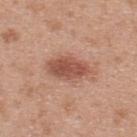notes = catalogued during a skin exam; not biopsied
patient = male, aged 28 to 32
automated metrics = a lesion color around L≈52 a*≈24 b*≈29 in CIELAB, about 12 CIELAB-L* units darker than the surrounding skin, and a normalized lesion–skin contrast near 8.5; radial color variation of about 1.5; an automated nevus-likeness rating near 100 out of 100 and a detector confidence of about 100 out of 100 that the crop contains a lesion
size = about 5 mm
tile lighting = white-light illumination
body site = the upper back
imaging modality = total-body-photography crop, ~15 mm field of view